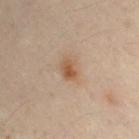workup: imaged on a skin check; not biopsied
imaging modality: 15 mm crop, total-body photography
diameter: ≈2.5 mm
site: the left upper arm
image-analysis metrics: a lesion area of about 4.5 mm², an outline eccentricity of about 0.7 (0 = round, 1 = elongated), and a shape-asymmetry score of about 0.15 (0 = symmetric); an average lesion color of about L≈44 a*≈15 b*≈27 (CIELAB), about 8 CIELAB-L* units darker than the surrounding skin, and a normalized lesion–skin contrast near 7.5; a border-irregularity index near 1.5/10, internal color variation of about 3.5 on a 0–10 scale, and peripheral color asymmetry of about 1
patient: male, approximately 30 years of age
tile lighting: cross-polarized